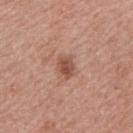biopsy status: catalogued during a skin exam; not biopsied | subject: male, aged approximately 60 | site: the right upper arm | image: ~15 mm tile from a whole-body skin photo.